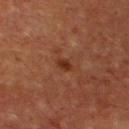{"biopsy_status": "not biopsied; imaged during a skin examination", "lighting": "cross-polarized", "site": "chest", "image": {"source": "total-body photography crop", "field_of_view_mm": 15}, "lesion_size": {"long_diameter_mm_approx": 2.0}, "automated_metrics": {"border_irregularity_0_10": 2.0, "peripheral_color_asymmetry": 1.0}, "patient": {"sex": "male", "age_approx": 65}}